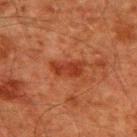{"biopsy_status": "not biopsied; imaged during a skin examination", "site": "upper back", "patient": {"sex": "male", "age_approx": 60}, "image": {"source": "total-body photography crop", "field_of_view_mm": 15}}